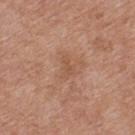{"biopsy_status": "not biopsied; imaged during a skin examination", "site": "upper back", "patient": {"sex": "female", "age_approx": 40}, "lesion_size": {"long_diameter_mm_approx": 2.5}, "image": {"source": "total-body photography crop", "field_of_view_mm": 15}}